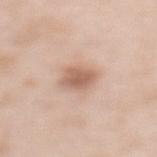<record>
<image>
  <source>total-body photography crop</source>
  <field_of_view_mm>15</field_of_view_mm>
</image>
<lesion_size>
  <long_diameter_mm_approx>3.5</long_diameter_mm_approx>
</lesion_size>
<automated_metrics>
  <area_mm2_approx>8.5</area_mm2_approx>
  <shape_asymmetry>0.15</shape_asymmetry>
  <vs_skin_contrast_norm>7.0</vs_skin_contrast_norm>
</automated_metrics>
<lighting>white-light</lighting>
<patient>
  <sex>female</sex>
  <age_approx>50</age_approx>
</patient>
<site>back</site>
</record>